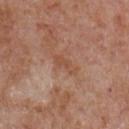notes: no biopsy performed (imaged during a skin exam)
subject: male, aged 63–67
site: the chest
image source: ~15 mm tile from a whole-body skin photo
automated metrics: a footprint of about 3.5 mm², a shape eccentricity near 0.9, and a symmetry-axis asymmetry near 0.45; an average lesion color of about L≈50 a*≈22 b*≈31 (CIELAB), a lesion–skin lightness drop of about 6, and a lesion-to-skin contrast of about 5.5 (normalized; higher = more distinct); a border-irregularity rating of about 5/10, a within-lesion color-variation index near 0.5/10, and a peripheral color-asymmetry measure near 0; a nevus-likeness score of about 0/100 and lesion-presence confidence of about 100/100
lesion size: ~3.5 mm (longest diameter)
tile lighting: white-light